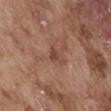The lesion was photographed on a routine skin check and not biopsied; there is no pathology result. The lesion is located on the abdomen. A male subject, approximately 75 years of age. Measured at roughly 2.5 mm in maximum diameter. Cropped from a total-body skin-imaging series; the visible field is about 15 mm.